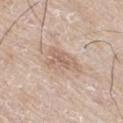Cropped from a whole-body photographic skin survey; the tile spans about 15 mm.
The lesion is located on the right thigh.
A male subject, about 60 years old.
An algorithmic analysis of the crop reported a color-variation rating of about 3/10 and peripheral color asymmetry of about 1.